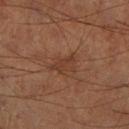The lesion was photographed on a routine skin check and not biopsied; there is no pathology result. A male subject aged approximately 65. The total-body-photography lesion software estimated a border-irregularity rating of about 3/10, a within-lesion color-variation index near 1.5/10, and peripheral color asymmetry of about 0.5. The lesion is on the left lower leg. A lesion tile, about 15 mm wide, cut from a 3D total-body photograph. About 3.5 mm across.